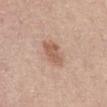Clinical impression: Captured during whole-body skin photography for melanoma surveillance; the lesion was not biopsied. Image and clinical context: A 15 mm crop from a total-body photograph taken for skin-cancer surveillance. A female subject approximately 60 years of age. From the abdomen.